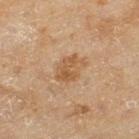Findings:
* workup — imaged on a skin check; not biopsied
* image-analysis metrics — an area of roughly 8 mm², a shape eccentricity near 0.7, and a shape-asymmetry score of about 0.25 (0 = symmetric); a lesion color around L≈51 a*≈18 b*≈33 in CIELAB, a lesion–skin lightness drop of about 8, and a normalized border contrast of about 6.5; border irregularity of about 2.5 on a 0–10 scale and peripheral color asymmetry of about 1.5
* acquisition — ~15 mm tile from a whole-body skin photo
* patient — female, aged approximately 60
* site — the leg
* tile lighting — cross-polarized